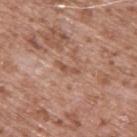Part of a total-body skin-imaging series; this lesion was reviewed on a skin check and was not flagged for biopsy.
Located on the upper back.
Imaged with white-light lighting.
The patient is a male in their mid-50s.
A lesion tile, about 15 mm wide, cut from a 3D total-body photograph.
The total-body-photography lesion software estimated a footprint of about 2 mm², an outline eccentricity of about 0.95 (0 = round, 1 = elongated), and two-axis asymmetry of about 0.55. And it measured a lesion color around L≈52 a*≈22 b*≈29 in CIELAB and about 9 CIELAB-L* units darker than the surrounding skin. The analysis additionally found a border-irregularity index near 6/10, a within-lesion color-variation index near 0/10, and peripheral color asymmetry of about 0. It also reported lesion-presence confidence of about 90/100.
The recorded lesion diameter is about 3 mm.